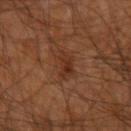Assessment: This lesion was catalogued during total-body skin photography and was not selected for biopsy. Clinical summary: From the right upper arm. A roughly 15 mm field-of-view crop from a total-body skin photograph. The patient is a male aged 43 to 47. Automated image analysis of the tile measured an area of roughly 6 mm², an eccentricity of roughly 0.7, and two-axis asymmetry of about 0.4. The software also gave about 6 CIELAB-L* units darker than the surrounding skin. The software also gave an automated nevus-likeness rating near 5 out of 100 and lesion-presence confidence of about 100/100.